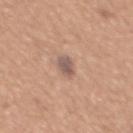{"biopsy_status": "not biopsied; imaged during a skin examination"}